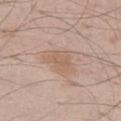Q: Was this lesion biopsied?
A: imaged on a skin check; not biopsied
Q: Automated lesion metrics?
A: an outline eccentricity of about 0.55 (0 = round, 1 = elongated); internal color variation of about 2 on a 0–10 scale and peripheral color asymmetry of about 0.5; a nevus-likeness score of about 0/100 and a lesion-detection confidence of about 100/100
Q: Patient demographics?
A: male, aged 48 to 52
Q: What kind of image is this?
A: ~15 mm crop, total-body skin-cancer survey
Q: Lesion size?
A: about 3 mm
Q: Where on the body is the lesion?
A: the left thigh